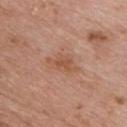Imaged during a routine full-body skin examination; the lesion was not biopsied and no histopathology is available.
The recorded lesion diameter is about 4.5 mm.
The total-body-photography lesion software estimated a footprint of about 6.5 mm², a shape eccentricity near 0.85, and two-axis asymmetry of about 0.3. The analysis additionally found roughly 7 lightness units darker than nearby skin and a normalized lesion–skin contrast near 6. The analysis additionally found a border-irregularity index near 4/10, a color-variation rating of about 2.5/10, and a peripheral color-asymmetry measure near 1. And it measured a classifier nevus-likeness of about 0/100 and a lesion-detection confidence of about 100/100.
The lesion is located on the chest.
A lesion tile, about 15 mm wide, cut from a 3D total-body photograph.
The subject is a male aged around 55.
The tile uses white-light illumination.